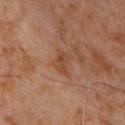Findings:
– biopsy status: catalogued during a skin exam; not biopsied
– lighting: cross-polarized illumination
– subject: male, about 60 years old
– site: the front of the torso
– size: about 2.5 mm
– acquisition: 15 mm crop, total-body photography
– automated metrics: an eccentricity of roughly 0.85; a lesion color around L≈41 a*≈21 b*≈32 in CIELAB, about 7 CIELAB-L* units darker than the surrounding skin, and a normalized lesion–skin contrast near 6.5; border irregularity of about 3 on a 0–10 scale and radial color variation of about 0.5; a classifier nevus-likeness of about 0/100 and a detector confidence of about 100 out of 100 that the crop contains a lesion